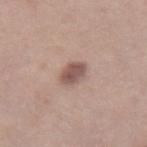This lesion was catalogued during total-body skin photography and was not selected for biopsy. This image is a 15 mm lesion crop taken from a total-body photograph. The subject is a female roughly 40 years of age. Located on the abdomen.